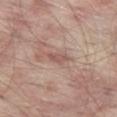Notes:
- workup — catalogued during a skin exam; not biopsied
- location — the left thigh
- acquisition — total-body-photography crop, ~15 mm field of view
- subject — male, aged approximately 65
- illumination — white-light illumination
- diameter — about 2.5 mm